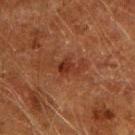Assessment: This lesion was catalogued during total-body skin photography and was not selected for biopsy. Acquisition and patient details: About 4 mm across. On the right upper arm. Imaged with cross-polarized lighting. This image is a 15 mm lesion crop taken from a total-body photograph. A male patient aged 58–62.